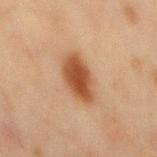Imaged during a routine full-body skin examination; the lesion was not biopsied and no histopathology is available. A male subject in their mid-40s. The tile uses cross-polarized illumination. The lesion's longest dimension is about 5 mm. The lesion is located on the mid back. A region of skin cropped from a whole-body photographic capture, roughly 15 mm wide.